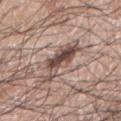notes: catalogued during a skin exam; not biopsied | image source: 15 mm crop, total-body photography | image-analysis metrics: an eccentricity of roughly 0.85 and a shape-asymmetry score of about 0.45 (0 = symmetric); a color-variation rating of about 8.5/10 and radial color variation of about 3; an automated nevus-likeness rating near 20 out of 100 and a detector confidence of about 95 out of 100 that the crop contains a lesion | lighting: white-light illumination | subject: male, aged 68 to 72 | lesion diameter: ≈5.5 mm | site: the left arm.